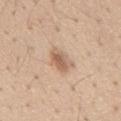Background:
Longest diameter approximately 3.5 mm. The patient is a male in their mid- to late 50s. The lesion is located on the mid back. The lesion-visualizer software estimated a lesion area of about 6 mm², an outline eccentricity of about 0.8 (0 = round, 1 = elongated), and a shape-asymmetry score of about 0.25 (0 = symmetric). And it measured a mean CIELAB color near L≈61 a*≈18 b*≈30, about 11 CIELAB-L* units darker than the surrounding skin, and a normalized lesion–skin contrast near 7. And it measured a detector confidence of about 100 out of 100 that the crop contains a lesion. Captured under white-light illumination. A close-up tile cropped from a whole-body skin photograph, about 15 mm across.